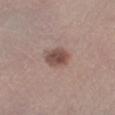Captured during whole-body skin photography for melanoma surveillance; the lesion was not biopsied. Captured under white-light illumination. Cropped from a whole-body photographic skin survey; the tile spans about 15 mm. A female patient, aged 28 to 32. Longest diameter approximately 3.5 mm. Located on the right lower leg.